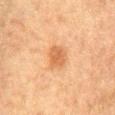<tbp_lesion>
<biopsy_status>not biopsied; imaged during a skin examination</biopsy_status>
<image>
  <source>total-body photography crop</source>
  <field_of_view_mm>15</field_of_view_mm>
</image>
<site>lower back</site>
<patient>
  <sex>male</sex>
  <age_approx>75</age_approx>
</patient>
<lighting>cross-polarized</lighting>
<lesion_size>
  <long_diameter_mm_approx>3.5</long_diameter_mm_approx>
</lesion_size>
</tbp_lesion>